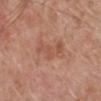The lesion was tiled from a total-body skin photograph and was not biopsied. A male patient aged 78–82. The recorded lesion diameter is about 5 mm. The lesion is on the leg. A region of skin cropped from a whole-body photographic capture, roughly 15 mm wide. An algorithmic analysis of the crop reported a lesion area of about 6.5 mm², an outline eccentricity of about 0.9 (0 = round, 1 = elongated), and two-axis asymmetry of about 0.5. The software also gave internal color variation of about 2.5 on a 0–10 scale and radial color variation of about 0.5. It also reported an automated nevus-likeness rating near 0 out of 100.